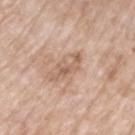The lesion was tiled from a total-body skin photograph and was not biopsied.
Approximately 4 mm at its widest.
From the right upper arm.
A close-up tile cropped from a whole-body skin photograph, about 15 mm across.
Imaged with white-light lighting.
A female subject aged 73 to 77.